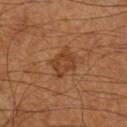* anatomic site: the left leg
* image source: ~15 mm crop, total-body skin-cancer survey
* illumination: cross-polarized illumination
* diameter: about 3.5 mm
* subject: male, aged 63–67